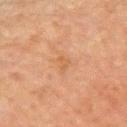Case summary:
- notes: imaged on a skin check; not biopsied
- size: ~2.5 mm (longest diameter)
- subject: male, roughly 65 years of age
- body site: the left upper arm
- image: ~15 mm crop, total-body skin-cancer survey
- automated lesion analysis: a lesion area of about 2.5 mm² and two-axis asymmetry of about 0.5; a mean CIELAB color near L≈48 a*≈19 b*≈32, about 5 CIELAB-L* units darker than the surrounding skin, and a lesion-to-skin contrast of about 5 (normalized; higher = more distinct); a border-irregularity rating of about 5/10, internal color variation of about 0 on a 0–10 scale, and radial color variation of about 0
- lighting: cross-polarized